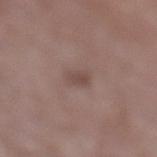follow-up = imaged on a skin check; not biopsied
diameter = ≈2.5 mm
body site = the left lower leg
patient = female, aged 68–72
acquisition = 15 mm crop, total-body photography